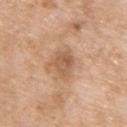<lesion>
<biopsy_status>not biopsied; imaged during a skin examination</biopsy_status>
<automated_metrics>
  <cielab_L>57</cielab_L>
  <cielab_a>20</cielab_a>
  <cielab_b>33</cielab_b>
  <vs_skin_darker_L>10.0</vs_skin_darker_L>
  <vs_skin_contrast_norm>6.5</vs_skin_contrast_norm>
  <border_irregularity_0_10>2.0</border_irregularity_0_10>
  <color_variation_0_10>4.0</color_variation_0_10>
  <peripheral_color_asymmetry>1.5</peripheral_color_asymmetry>
  <lesion_detection_confidence_0_100>100</lesion_detection_confidence_0_100>
</automated_metrics>
<image>
  <source>total-body photography crop</source>
  <field_of_view_mm>15</field_of_view_mm>
</image>
<patient>
  <sex>female</sex>
  <age_approx>75</age_approx>
</patient>
<lighting>white-light</lighting>
<lesion_size>
  <long_diameter_mm_approx>3.0</long_diameter_mm_approx>
</lesion_size>
<site>right upper arm</site>
</lesion>